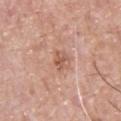{"biopsy_status": "not biopsied; imaged during a skin examination", "lesion_size": {"long_diameter_mm_approx": 2.5}, "lighting": "white-light", "image": {"source": "total-body photography crop", "field_of_view_mm": 15}, "patient": {"sex": "male", "age_approx": 55}}